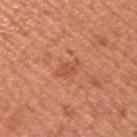Clinical summary:
The lesion is on the right upper arm. A 15 mm close-up extracted from a 3D total-body photography capture. The tile uses white-light illumination. A male patient, roughly 55 years of age.